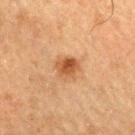Case summary:
– workup — no biopsy performed (imaged during a skin exam)
– image source — 15 mm crop, total-body photography
– image-analysis metrics — an area of roughly 6 mm², an eccentricity of roughly 0.55, and two-axis asymmetry of about 0.2; a within-lesion color-variation index near 4.5/10 and radial color variation of about 1.5
– diameter — ≈3 mm
– site — the right thigh
– tile lighting — cross-polarized illumination
– subject — male, about 75 years old